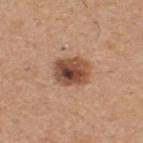  biopsy_status: not biopsied; imaged during a skin examination
  site: upper back
  image:
    source: total-body photography crop
    field_of_view_mm: 15
  lighting: white-light
  automated_metrics:
    eccentricity: 0.6
    shape_asymmetry: 0.1
    border_irregularity_0_10: 1.0
    color_variation_0_10: 10.0
    peripheral_color_asymmetry: 3.5
    lesion_detection_confidence_0_100: 100
  patient:
    sex: male
    age_approx: 60